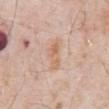biopsy status: total-body-photography surveillance lesion; no biopsy | anatomic site: the abdomen | tile lighting: white-light | acquisition: total-body-photography crop, ~15 mm field of view | patient: male, roughly 80 years of age | diameter: ≈4 mm.